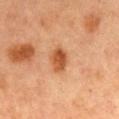{
  "biopsy_status": "not biopsied; imaged during a skin examination",
  "patient": {
    "sex": "female",
    "age_approx": 60
  },
  "site": "chest",
  "image": {
    "source": "total-body photography crop",
    "field_of_view_mm": 15
  },
  "automated_metrics": {
    "shape_asymmetry": 0.15,
    "vs_skin_darker_L": 12.0,
    "vs_skin_contrast_norm": 9.5,
    "border_irregularity_0_10": 1.0,
    "color_variation_0_10": 3.5,
    "peripheral_color_asymmetry": 1.5
  },
  "lighting": "cross-polarized",
  "lesion_size": {
    "long_diameter_mm_approx": 3.0
  }
}